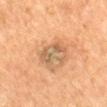The lesion was photographed on a routine skin check and not biopsied; there is no pathology result. The patient is a female aged 58 to 62. A close-up tile cropped from a whole-body skin photograph, about 15 mm across. This is a cross-polarized tile. Located on the mid back.